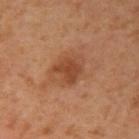Assessment:
The lesion was tiled from a total-body skin photograph and was not biopsied.
Image and clinical context:
Located on the right upper arm. Captured under cross-polarized illumination. Measured at roughly 3.5 mm in maximum diameter. This image is a 15 mm lesion crop taken from a total-body photograph. The patient is a female aged 38 to 42.